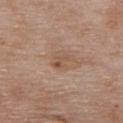Clinical impression: Imaged during a routine full-body skin examination; the lesion was not biopsied and no histopathology is available. Background: This image is a 15 mm lesion crop taken from a total-body photograph. The lesion is located on the chest. A male subject about 80 years old.